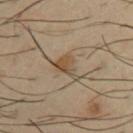{"biopsy_status": "not biopsied; imaged during a skin examination", "site": "left upper arm", "lighting": "cross-polarized", "image": {"source": "total-body photography crop", "field_of_view_mm": 15}, "automated_metrics": {"eccentricity": 0.7, "cielab_L": 47, "cielab_a": 13, "cielab_b": 30, "vs_skin_contrast_norm": 7.5, "nevus_likeness_0_100": 80, "lesion_detection_confidence_0_100": 100}, "lesion_size": {"long_diameter_mm_approx": 3.5}, "patient": {"sex": "male", "age_approx": 40}}